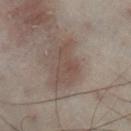workup = total-body-photography surveillance lesion; no biopsy
location = the left leg
lesion size = ≈5 mm
automated metrics = a lesion color around L≈38 a*≈11 b*≈18 in CIELAB, roughly 6 lightness units darker than nearby skin, and a normalized border contrast of about 5.5; a border-irregularity index near 5.5/10, a within-lesion color-variation index near 2.5/10, and a peripheral color-asymmetry measure near 1
imaging modality = ~15 mm tile from a whole-body skin photo
subject = male, approximately 50 years of age
tile lighting = cross-polarized illumination